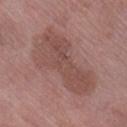Assessment: The lesion was tiled from a total-body skin photograph and was not biopsied. Acquisition and patient details: A close-up tile cropped from a whole-body skin photograph, about 15 mm across. Measured at roughly 9 mm in maximum diameter. The tile uses white-light illumination. Located on the right lower leg. A female subject aged approximately 70. An algorithmic analysis of the crop reported a footprint of about 28 mm², a shape eccentricity near 0.9, and two-axis asymmetry of about 0.3. The software also gave a border-irregularity rating of about 5/10, a color-variation rating of about 3/10, and a peripheral color-asymmetry measure near 1. The software also gave a lesion-detection confidence of about 100/100.